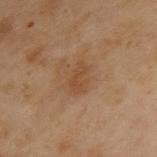workup: total-body-photography surveillance lesion; no biopsy | acquisition: total-body-photography crop, ~15 mm field of view | lesion size: ≈3.5 mm | lighting: cross-polarized illumination | subject: male, approximately 55 years of age | body site: the upper back.